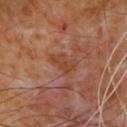This lesion was catalogued during total-body skin photography and was not selected for biopsy. Imaged with cross-polarized lighting. From the upper back. Automated image analysis of the tile measured a lesion area of about 5 mm² and a symmetry-axis asymmetry near 0.4. And it measured an average lesion color of about L≈40 a*≈23 b*≈29 (CIELAB), roughly 6 lightness units darker than nearby skin, and a lesion-to-skin contrast of about 6 (normalized; higher = more distinct). And it measured an automated nevus-likeness rating near 0 out of 100 and a lesion-detection confidence of about 100/100. A lesion tile, about 15 mm wide, cut from a 3D total-body photograph. About 3.5 mm across. The patient is a male about 60 years old.